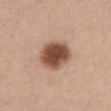Captured during whole-body skin photography for melanoma surveillance; the lesion was not biopsied. A 15 mm close-up tile from a total-body photography series done for melanoma screening. A female patient, roughly 45 years of age. The lesion is on the abdomen. Measured at roughly 4.5 mm in maximum diameter. The tile uses white-light illumination.